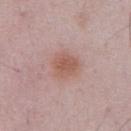Notes:
– workup · catalogued during a skin exam; not biopsied
– image · ~15 mm tile from a whole-body skin photo
– automated lesion analysis · a lesion color around L≈56 a*≈21 b*≈26 in CIELAB, a lesion–skin lightness drop of about 8, and a normalized border contrast of about 7; an automated nevus-likeness rating near 90 out of 100 and a lesion-detection confidence of about 100/100
– patient · male, approximately 50 years of age
– site · the chest
– illumination · white-light illumination
– lesion diameter · ≈3 mm